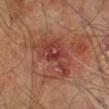- biopsy status · no biopsy performed (imaged during a skin exam)
- diameter · ≈6 mm
- patient · male, about 60 years old
- body site · the right leg
- image · total-body-photography crop, ~15 mm field of view
- lighting · cross-polarized illumination